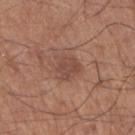Q: Who is the patient?
A: male, aged approximately 65
Q: What is the anatomic site?
A: the right upper arm
Q: What is the lesion's diameter?
A: ≈3 mm
Q: What is the imaging modality?
A: ~15 mm crop, total-body skin-cancer survey
Q: How was the tile lit?
A: white-light